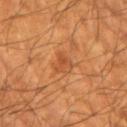Clinical impression: This lesion was catalogued during total-body skin photography and was not selected for biopsy. Background: Measured at roughly 2.5 mm in maximum diameter. From the right forearm. The lesion-visualizer software estimated a mean CIELAB color near L≈47 a*≈26 b*≈38, a lesion–skin lightness drop of about 7, and a normalized lesion–skin contrast near 5.5. A male patient, approximately 65 years of age. Cropped from a whole-body photographic skin survey; the tile spans about 15 mm. The tile uses cross-polarized illumination.